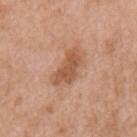{"patient": {"sex": "male", "age_approx": 75}, "lighting": "white-light", "lesion_size": {"long_diameter_mm_approx": 5.0}, "site": "mid back", "image": {"source": "total-body photography crop", "field_of_view_mm": 15}}